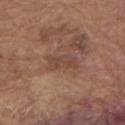<lesion>
<biopsy_status>not biopsied; imaged during a skin examination</biopsy_status>
</lesion>